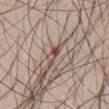Q: Was this lesion biopsied?
A: catalogued during a skin exam; not biopsied
Q: What is the lesion's diameter?
A: ≈4 mm
Q: What are the patient's age and sex?
A: male, aged 23–27
Q: Automated lesion metrics?
A: an area of roughly 4.5 mm², an eccentricity of roughly 0.95, and two-axis asymmetry of about 0.45; a within-lesion color-variation index near 9.5/10 and a peripheral color-asymmetry measure near 3.5; a classifier nevus-likeness of about 0/100 and a lesion-detection confidence of about 90/100
Q: Where on the body is the lesion?
A: the front of the torso
Q: What kind of image is this?
A: total-body-photography crop, ~15 mm field of view
Q: How was the tile lit?
A: white-light